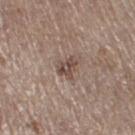This lesion was catalogued during total-body skin photography and was not selected for biopsy. Automated image analysis of the tile measured a classifier nevus-likeness of about 0/100 and a lesion-detection confidence of about 100/100. The subject is a female approximately 65 years of age. Captured under white-light illumination. The lesion is on the right thigh. A roughly 15 mm field-of-view crop from a total-body skin photograph.